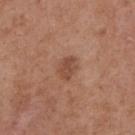Clinical impression: This lesion was catalogued during total-body skin photography and was not selected for biopsy. Image and clinical context: Cropped from a total-body skin-imaging series; the visible field is about 15 mm. On the upper back. The patient is a female roughly 40 years of age. Longest diameter approximately 2.5 mm. Imaged with white-light lighting. Automated image analysis of the tile measured an outline eccentricity of about 0.7 (0 = round, 1 = elongated). The analysis additionally found a lesion color around L≈47 a*≈22 b*≈30 in CIELAB, roughly 9 lightness units darker than nearby skin, and a normalized border contrast of about 7.